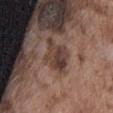Imaged during a routine full-body skin examination; the lesion was not biopsied and no histopathology is available.
The lesion is on the abdomen.
A 15 mm close-up extracted from a 3D total-body photography capture.
The patient is a male aged approximately 75.
Longest diameter approximately 4.5 mm.